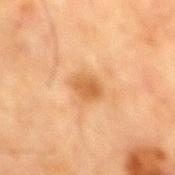Impression:
No biopsy was performed on this lesion — it was imaged during a full skin examination and was not determined to be concerning.
Background:
The lesion is on the back. A male subject, approximately 65 years of age. A 15 mm crop from a total-body photograph taken for skin-cancer surveillance.